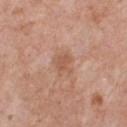Findings:
– workup: catalogued during a skin exam; not biopsied